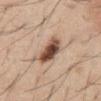Findings:
• biopsy status: total-body-photography surveillance lesion; no biopsy
• imaging modality: ~15 mm tile from a whole-body skin photo
• subject: male, in their mid-40s
• automated metrics: a footprint of about 9 mm² and a symmetry-axis asymmetry near 0.2; a border-irregularity index near 2/10 and internal color variation of about 9 on a 0–10 scale
• size: ~4 mm (longest diameter)
• anatomic site: the abdomen
• tile lighting: white-light illumination